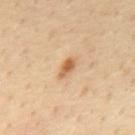Clinical impression: The lesion was photographed on a routine skin check and not biopsied; there is no pathology result. Clinical summary: Cropped from a total-body skin-imaging series; the visible field is about 15 mm. Located on the mid back. The patient is a male aged approximately 55. Automated image analysis of the tile measured a footprint of about 3 mm², an eccentricity of roughly 0.85, and a symmetry-axis asymmetry near 0.2. And it measured a classifier nevus-likeness of about 85/100 and lesion-presence confidence of about 100/100. This is a cross-polarized tile. Longest diameter approximately 3 mm.